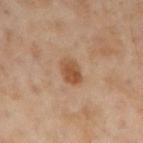A close-up tile cropped from a whole-body skin photograph, about 15 mm across. Located on the right upper arm. A male subject aged around 55.